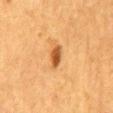{
  "biopsy_status": "not biopsied; imaged during a skin examination",
  "site": "mid back",
  "patient": {
    "sex": "female",
    "age_approx": 55
  },
  "lesion_size": {
    "long_diameter_mm_approx": 3.0
  },
  "image": {
    "source": "total-body photography crop",
    "field_of_view_mm": 15
  }
}